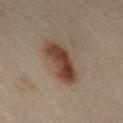<case>
<biopsy_status>not biopsied; imaged during a skin examination</biopsy_status>
<patient>
  <sex>female</sex>
  <age_approx>40</age_approx>
</patient>
<lesion_size>
  <long_diameter_mm_approx>6.0</long_diameter_mm_approx>
</lesion_size>
<image>
  <source>total-body photography crop</source>
  <field_of_view_mm>15</field_of_view_mm>
</image>
<site>arm</site>
<lighting>cross-polarized</lighting>
<automated_metrics>
  <cielab_L>43</cielab_L>
  <cielab_a>18</cielab_a>
  <cielab_b>27</cielab_b>
  <vs_skin_darker_L>13.0</vs_skin_darker_L>
  <vs_skin_contrast_norm>10.0</vs_skin_contrast_norm>
  <border_irregularity_0_10>2.5</border_irregularity_0_10>
  <peripheral_color_asymmetry>2.5</peripheral_color_asymmetry>
  <nevus_likeness_0_100>100</nevus_likeness_0_100>
  <lesion_detection_confidence_0_100>100</lesion_detection_confidence_0_100>
</automated_metrics>
</case>